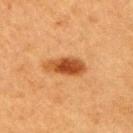{"automated_metrics": {"nevus_likeness_0_100": 100, "lesion_detection_confidence_0_100": 100}, "image": {"source": "total-body photography crop", "field_of_view_mm": 15}, "lesion_size": {"long_diameter_mm_approx": 5.0}, "site": "right upper arm", "patient": {"sex": "female", "age_approx": 40}}